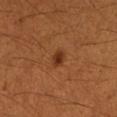<tbp_lesion>
  <biopsy_status>not biopsied; imaged during a skin examination</biopsy_status>
  <patient>
    <sex>female</sex>
    <age_approx>40</age_approx>
  </patient>
  <automated_metrics>
    <cielab_L>34</cielab_L>
    <cielab_a>25</cielab_a>
    <cielab_b>34</cielab_b>
    <vs_skin_darker_L>10.0</vs_skin_darker_L>
    <vs_skin_contrast_norm>9.0</vs_skin_contrast_norm>
    <nevus_likeness_0_100>95</nevus_likeness_0_100>
    <lesion_detection_confidence_0_100>100</lesion_detection_confidence_0_100>
  </automated_metrics>
  <lighting>cross-polarized</lighting>
  <image>
    <source>total-body photography crop</source>
    <field_of_view_mm>15</field_of_view_mm>
  </image>
  <site>left forearm</site>
</tbp_lesion>